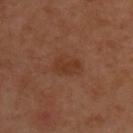Q: Is there a histopathology result?
A: imaged on a skin check; not biopsied
Q: What is the imaging modality?
A: ~15 mm tile from a whole-body skin photo
Q: What did automated image analysis measure?
A: a mean CIELAB color near L≈35 a*≈22 b*≈30, about 6 CIELAB-L* units darker than the surrounding skin, and a lesion-to-skin contrast of about 6 (normalized; higher = more distinct); a border-irregularity index near 2.5/10 and a color-variation rating of about 2/10
Q: How was the tile lit?
A: cross-polarized
Q: Who is the patient?
A: male, roughly 50 years of age
Q: Lesion location?
A: the upper back
Q: Lesion size?
A: about 3.5 mm